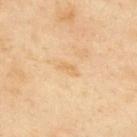Impression:
Part of a total-body skin-imaging series; this lesion was reviewed on a skin check and was not flagged for biopsy.
Background:
Cropped from a total-body skin-imaging series; the visible field is about 15 mm. The lesion is located on the upper back. Captured under cross-polarized illumination. A male subject, about 55 years old.